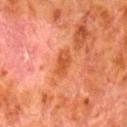Q: Is there a histopathology result?
A: imaged on a skin check; not biopsied
Q: Lesion location?
A: the right lower leg
Q: How was this image acquired?
A: total-body-photography crop, ~15 mm field of view
Q: What is the lesion's diameter?
A: about 3 mm
Q: Who is the patient?
A: male, in their 80s
Q: What lighting was used for the tile?
A: cross-polarized illumination